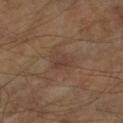{
  "biopsy_status": "not biopsied; imaged during a skin examination",
  "site": "right forearm",
  "automated_metrics": {
    "border_irregularity_0_10": 3.5,
    "color_variation_0_10": 2.0
  },
  "lighting": "cross-polarized",
  "image": {
    "source": "total-body photography crop",
    "field_of_view_mm": 15
  },
  "patient": {
    "sex": "male",
    "age_approx": 70
  }
}